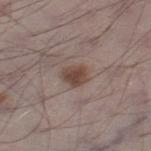workup: catalogued during a skin exam; not biopsied | patient: male, approximately 70 years of age | anatomic site: the right thigh | image source: total-body-photography crop, ~15 mm field of view | lesion size: ~3 mm (longest diameter).